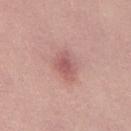The lesion was photographed on a routine skin check and not biopsied; there is no pathology result. The patient is a male aged 28 to 32. Imaged with white-light lighting. Cropped from a whole-body photographic skin survey; the tile spans about 15 mm. About 4 mm across.